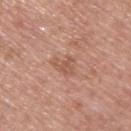{"biopsy_status": "not biopsied; imaged during a skin examination"}